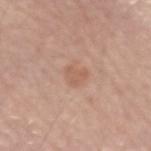Impression:
Imaged during a routine full-body skin examination; the lesion was not biopsied and no histopathology is available.
Background:
Measured at roughly 3 mm in maximum diameter. Automated tile analysis of the lesion measured a lesion color around L≈59 a*≈21 b*≈29 in CIELAB and a normalized border contrast of about 5. Located on the right upper arm. The tile uses white-light illumination. A male patient aged 53 to 57. A 15 mm crop from a total-body photograph taken for skin-cancer surveillance.